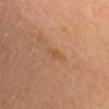This lesion was catalogued during total-body skin photography and was not selected for biopsy. Imaged with cross-polarized lighting. A female patient, roughly 35 years of age. On the head or neck. A region of skin cropped from a whole-body photographic capture, roughly 15 mm wide.